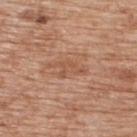follow-up: imaged on a skin check; not biopsied
imaging modality: 15 mm crop, total-body photography
subject: male, approximately 60 years of age
illumination: white-light illumination
body site: the upper back
TBP lesion metrics: a footprint of about 5 mm² and a shape eccentricity near 0.85; a mean CIELAB color near L≈54 a*≈22 b*≈32, roughly 7 lightness units darker than nearby skin, and a normalized lesion–skin contrast near 5; a border-irregularity rating of about 6.5/10, internal color variation of about 1.5 on a 0–10 scale, and radial color variation of about 0.5; an automated nevus-likeness rating near 0 out of 100
lesion diameter: ~4 mm (longest diameter)